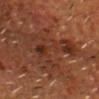Q: Lesion size?
A: about 7 mm
Q: Patient demographics?
A: male, aged 53–57
Q: Illumination type?
A: cross-polarized
Q: What is the imaging modality?
A: total-body-photography crop, ~15 mm field of view
Q: What is the anatomic site?
A: the head or neck